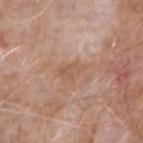Assessment:
Imaged during a routine full-body skin examination; the lesion was not biopsied and no histopathology is available.
Background:
A male patient approximately 75 years of age. This is a white-light tile. The recorded lesion diameter is about 3.5 mm. From the right forearm. A region of skin cropped from a whole-body photographic capture, roughly 15 mm wide.